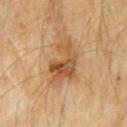Findings:
– notes: imaged on a skin check; not biopsied
– acquisition: total-body-photography crop, ~15 mm field of view
– diameter: about 5.5 mm
– subject: male, about 60 years old
– tile lighting: cross-polarized illumination
– site: the abdomen